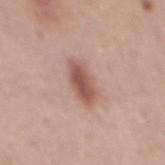Q: Was a biopsy performed?
A: imaged on a skin check; not biopsied
Q: Who is the patient?
A: male, in their mid- to late 40s
Q: What kind of image is this?
A: ~15 mm tile from a whole-body skin photo
Q: Lesion location?
A: the mid back